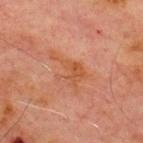Recorded during total-body skin imaging; not selected for excision or biopsy. A male patient roughly 70 years of age. From the chest. A roughly 15 mm field-of-view crop from a total-body skin photograph.